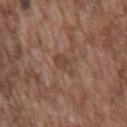Impression:
Part of a total-body skin-imaging series; this lesion was reviewed on a skin check and was not flagged for biopsy.
Context:
Approximately 3 mm at its widest. This is a white-light tile. The subject is a male approximately 75 years of age. A 15 mm crop from a total-body photograph taken for skin-cancer surveillance. On the chest.